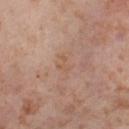image:
  source: total-body photography crop
  field_of_view_mm: 15
lighting: cross-polarized
site: leg
patient:
  sex: female
  age_approx: 55